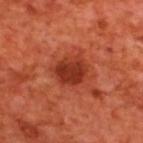- biopsy status · imaged on a skin check; not biopsied
- location · the upper back
- image source · 15 mm crop, total-body photography
- patient · male, aged around 70